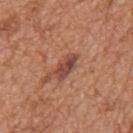Assessment:
Part of a total-body skin-imaging series; this lesion was reviewed on a skin check and was not flagged for biopsy.
Clinical summary:
The subject is a male approximately 65 years of age. Located on the mid back. A 15 mm close-up extracted from a 3D total-body photography capture.